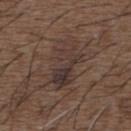Recorded during total-body skin imaging; not selected for excision or biopsy. Automated tile analysis of the lesion measured a lesion color around L≈34 a*≈14 b*≈19 in CIELAB, about 7 CIELAB-L* units darker than the surrounding skin, and a normalized border contrast of about 7.5. The software also gave a nevus-likeness score of about 0/100 and lesion-presence confidence of about 80/100. A male subject aged 48 to 52. The lesion's longest dimension is about 5.5 mm. This is a white-light tile. This image is a 15 mm lesion crop taken from a total-body photograph. Located on the upper back.